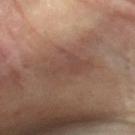| feature | finding |
|---|---|
| tile lighting | cross-polarized |
| image | 15 mm crop, total-body photography |
| TBP lesion metrics | an area of roughly 9.5 mm², an eccentricity of roughly 0.9, and a shape-asymmetry score of about 0.35 (0 = symmetric); a border-irregularity rating of about 5/10, internal color variation of about 2 on a 0–10 scale, and peripheral color asymmetry of about 0.5; a lesion-detection confidence of about 85/100 |
| patient | female, aged around 75 |
| site | the right forearm |
| lesion size | ≈5.5 mm |